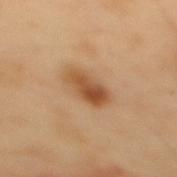Q: Is there a histopathology result?
A: no biopsy performed (imaged during a skin exam)
Q: How was this image acquired?
A: 15 mm crop, total-body photography
Q: Who is the patient?
A: male, about 55 years old
Q: Where on the body is the lesion?
A: the mid back